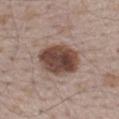No biopsy was performed on this lesion — it was imaged during a full skin examination and was not determined to be concerning.
Approximately 5.5 mm at its widest.
Automated image analysis of the tile measured a footprint of about 18 mm², an eccentricity of roughly 0.65, and a shape-asymmetry score of about 0.1 (0 = symmetric).
A male patient, aged around 65.
A 15 mm crop from a total-body photograph taken for skin-cancer surveillance.
Captured under white-light illumination.
Located on the mid back.